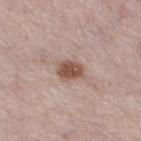  biopsy_status: not biopsied; imaged during a skin examination